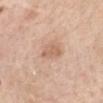Notes:
– biopsy status — imaged on a skin check; not biopsied
– automated lesion analysis — a lesion color around L≈63 a*≈20 b*≈31 in CIELAB, a lesion–skin lightness drop of about 9, and a normalized lesion–skin contrast near 6; a border-irregularity rating of about 2.5/10, internal color variation of about 2 on a 0–10 scale, and a peripheral color-asymmetry measure near 0.5; a nevus-likeness score of about 10/100
– acquisition — ~15 mm crop, total-body skin-cancer survey
– patient — female, about 65 years old
– body site — the front of the torso
– tile lighting — white-light illumination